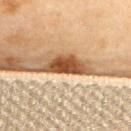Assessment:
Imaged during a routine full-body skin examination; the lesion was not biopsied and no histopathology is available.
Acquisition and patient details:
Automated tile analysis of the lesion measured an area of roughly 8 mm². The analysis additionally found about 21 CIELAB-L* units darker than the surrounding skin and a normalized border contrast of about 13.5. And it measured a color-variation rating of about 4.5/10 and a peripheral color-asymmetry measure near 1.5. A lesion tile, about 15 mm wide, cut from a 3D total-body photograph. Captured under cross-polarized illumination. On the upper back. About 5 mm across. A female subject, roughly 60 years of age.